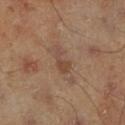This lesion was catalogued during total-body skin photography and was not selected for biopsy.
A 15 mm close-up extracted from a 3D total-body photography capture.
A male subject about 45 years old.
The lesion is on the leg.
About 3 mm across.
The lesion-visualizer software estimated a footprint of about 4 mm², an outline eccentricity of about 0.9 (0 = round, 1 = elongated), and a shape-asymmetry score of about 0.45 (0 = symmetric). The analysis additionally found a border-irregularity rating of about 5/10, a color-variation rating of about 0.5/10, and a peripheral color-asymmetry measure near 0. And it measured lesion-presence confidence of about 100/100.
This is a cross-polarized tile.